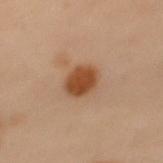notes — no biopsy performed (imaged during a skin exam)
automated metrics — an average lesion color of about L≈40 a*≈20 b*≈31 (CIELAB), roughly 12 lightness units darker than nearby skin, and a lesion-to-skin contrast of about 10.5 (normalized; higher = more distinct); a nevus-likeness score of about 100/100
acquisition — total-body-photography crop, ~15 mm field of view
site — the upper back
illumination — cross-polarized illumination
subject — female, approximately 60 years of age